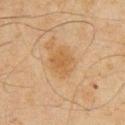Part of a total-body skin-imaging series; this lesion was reviewed on a skin check and was not flagged for biopsy.
A male subject, aged around 65.
Automated image analysis of the tile measured an area of roughly 9 mm², an outline eccentricity of about 0.55 (0 = round, 1 = elongated), and a shape-asymmetry score of about 0.15 (0 = symmetric). The software also gave an average lesion color of about L≈48 a*≈16 b*≈34 (CIELAB), about 6 CIELAB-L* units darker than the surrounding skin, and a normalized lesion–skin contrast near 6. The analysis additionally found a border-irregularity rating of about 1.5/10, internal color variation of about 2.5 on a 0–10 scale, and a peripheral color-asymmetry measure near 1. It also reported an automated nevus-likeness rating near 15 out of 100 and a detector confidence of about 100 out of 100 that the crop contains a lesion.
Captured under cross-polarized illumination.
On the chest.
The lesion's longest dimension is about 3.5 mm.
A region of skin cropped from a whole-body photographic capture, roughly 15 mm wide.